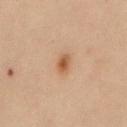Assessment:
Part of a total-body skin-imaging series; this lesion was reviewed on a skin check and was not flagged for biopsy.
Background:
Imaged with cross-polarized lighting. A female patient, aged 28 to 32. The lesion is on the mid back. A 15 mm close-up tile from a total-body photography series done for melanoma screening.